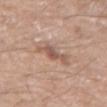| field | value |
|---|---|
| biopsy status | total-body-photography surveillance lesion; no biopsy |
| patient | male, aged around 70 |
| image | ~15 mm crop, total-body skin-cancer survey |
| illumination | white-light illumination |
| automated lesion analysis | a footprint of about 7 mm², an outline eccentricity of about 0.8 (0 = round, 1 = elongated), and two-axis asymmetry of about 0.4; internal color variation of about 4 on a 0–10 scale and a peripheral color-asymmetry measure near 1; a nevus-likeness score of about 0/100 and a lesion-detection confidence of about 100/100 |
| location | the arm |
| diameter | ~4.5 mm (longest diameter) |